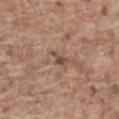Notes:
- notes — no biopsy performed (imaged during a skin exam)
- lighting — white-light illumination
- image-analysis metrics — a lesion color around L≈48 a*≈19 b*≈26 in CIELAB and a lesion-to-skin contrast of about 7.5 (normalized; higher = more distinct); a border-irregularity index near 5.5/10, a within-lesion color-variation index near 0/10, and radial color variation of about 0; a nevus-likeness score of about 0/100 and lesion-presence confidence of about 75/100
- anatomic site — the lower back
- subject — male, aged 78–82
- acquisition — ~15 mm tile from a whole-body skin photo
- size — ~2.5 mm (longest diameter)